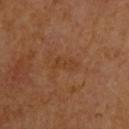Assessment:
The lesion was photographed on a routine skin check and not biopsied; there is no pathology result.
Acquisition and patient details:
The recorded lesion diameter is about 3 mm. Automated tile analysis of the lesion measured a nevus-likeness score of about 0/100 and a lesion-detection confidence of about 100/100. A lesion tile, about 15 mm wide, cut from a 3D total-body photograph. The tile uses cross-polarized illumination. From the arm. A male patient, aged 63–67.